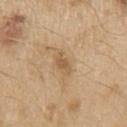Impression: The lesion was tiled from a total-body skin photograph and was not biopsied. Acquisition and patient details: The patient is a male about 70 years old. The lesion-visualizer software estimated a lesion area of about 3 mm², a shape eccentricity near 0.8, and a shape-asymmetry score of about 0.35 (0 = symmetric). Captured under white-light illumination. The lesion is located on the left upper arm. Cropped from a whole-body photographic skin survey; the tile spans about 15 mm.